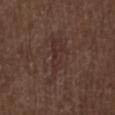The lesion was photographed on a routine skin check and not biopsied; there is no pathology result.
A male patient aged around 70.
On the back.
Captured under white-light illumination.
A 15 mm crop from a total-body photograph taken for skin-cancer surveillance.
The recorded lesion diameter is about 6.5 mm.